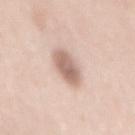Imaged during a routine full-body skin examination; the lesion was not biopsied and no histopathology is available.